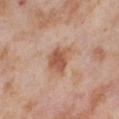Assessment:
Captured during whole-body skin photography for melanoma surveillance; the lesion was not biopsied.
Clinical summary:
From the right thigh. The subject is a female roughly 55 years of age. About 4 mm across. A 15 mm close-up extracted from a 3D total-body photography capture.